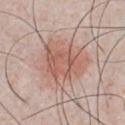This lesion was catalogued during total-body skin photography and was not selected for biopsy. The recorded lesion diameter is about 5.5 mm. The tile uses white-light illumination. A 15 mm close-up tile from a total-body photography series done for melanoma screening. From the chest. Automated image analysis of the tile measured a lesion area of about 19 mm², a shape eccentricity near 0.55, and a shape-asymmetry score of about 0.35 (0 = symmetric). The software also gave a border-irregularity rating of about 4.5/10, internal color variation of about 4.5 on a 0–10 scale, and radial color variation of about 1.5. The analysis additionally found a nevus-likeness score of about 90/100 and a detector confidence of about 100 out of 100 that the crop contains a lesion. The subject is a male aged approximately 25.